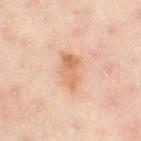Q: Was this lesion biopsied?
A: total-body-photography surveillance lesion; no biopsy
Q: Patient demographics?
A: female, roughly 55 years of age
Q: Lesion size?
A: ~4.5 mm (longest diameter)
Q: What is the imaging modality?
A: total-body-photography crop, ~15 mm field of view
Q: Lesion location?
A: the leg
Q: What did automated image analysis measure?
A: a shape eccentricity near 0.85 and a symmetry-axis asymmetry near 0.25; a detector confidence of about 100 out of 100 that the crop contains a lesion